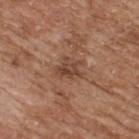Findings:
* notes — catalogued during a skin exam; not biopsied
* anatomic site — the upper back
* image source — ~15 mm crop, total-body skin-cancer survey
* diameter — ~3.5 mm (longest diameter)
* lighting — white-light illumination
* image-analysis metrics — a lesion area of about 7 mm² and two-axis asymmetry of about 0.45; an average lesion color of about L≈45 a*≈20 b*≈29 (CIELAB) and a normalized border contrast of about 7; border irregularity of about 5 on a 0–10 scale, a color-variation rating of about 4/10, and a peripheral color-asymmetry measure near 1.5
* subject — male, aged around 65